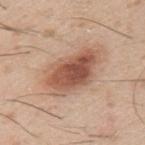This lesion was catalogued during total-body skin photography and was not selected for biopsy. Cropped from a whole-body photographic skin survey; the tile spans about 15 mm. Measured at roughly 6.5 mm in maximum diameter. A male subject, aged 28 to 32. The lesion is on the left upper arm. Imaged with white-light lighting.